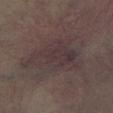- workup: no biopsy performed (imaged during a skin exam)
- image source: 15 mm crop, total-body photography
- patient: aged approximately 55
- location: the right lower leg